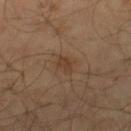This lesion was catalogued during total-body skin photography and was not selected for biopsy. Located on the right lower leg. This is a cross-polarized tile. The recorded lesion diameter is about 2.5 mm. The subject is a male about 65 years old. Automated image analysis of the tile measured border irregularity of about 3 on a 0–10 scale and a within-lesion color-variation index near 1/10. The analysis additionally found a nevus-likeness score of about 10/100. A region of skin cropped from a whole-body photographic capture, roughly 15 mm wide.